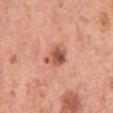No biopsy was performed on this lesion — it was imaged during a full skin examination and was not determined to be concerning. The total-body-photography lesion software estimated a border-irregularity index near 3.5/10, internal color variation of about 6.5 on a 0–10 scale, and peripheral color asymmetry of about 2. The software also gave an automated nevus-likeness rating near 55 out of 100 and a detector confidence of about 100 out of 100 that the crop contains a lesion. Located on the left upper arm. Cropped from a total-body skin-imaging series; the visible field is about 15 mm. This is a white-light tile. A female subject roughly 50 years of age. The recorded lesion diameter is about 3 mm.